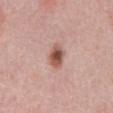follow-up = catalogued during a skin exam; not biopsied | lesion diameter = ~3 mm (longest diameter) | site = the abdomen | image source = total-body-photography crop, ~15 mm field of view | subject = male, aged 48–52 | lighting = white-light.